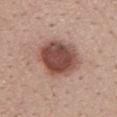Clinical impression: Part of a total-body skin-imaging series; this lesion was reviewed on a skin check and was not flagged for biopsy. Clinical summary: This image is a 15 mm lesion crop taken from a total-body photograph. On the upper back. Imaged with white-light lighting. Approximately 5.5 mm at its widest. Automated image analysis of the tile measured a lesion area of about 21 mm², a shape eccentricity near 0.6, and a shape-asymmetry score of about 0.15 (0 = symmetric). The analysis additionally found a border-irregularity rating of about 2/10 and a color-variation rating of about 5/10. A female subject, roughly 25 years of age.